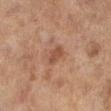Q: Was a biopsy performed?
A: catalogued during a skin exam; not biopsied